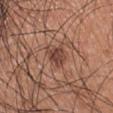Captured during whole-body skin photography for melanoma surveillance; the lesion was not biopsied. Imaged with white-light lighting. A male subject, in their mid- to late 30s. A lesion tile, about 15 mm wide, cut from a 3D total-body photograph. The lesion's longest dimension is about 3.5 mm. On the arm. Automated tile analysis of the lesion measured an outline eccentricity of about 0.7 (0 = round, 1 = elongated) and a symmetry-axis asymmetry near 0.3. The analysis additionally found internal color variation of about 3 on a 0–10 scale and a peripheral color-asymmetry measure near 1. The software also gave lesion-presence confidence of about 100/100.